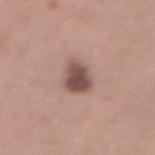biopsy status: total-body-photography surveillance lesion; no biopsy | automated lesion analysis: a mean CIELAB color near L≈50 a*≈19 b*≈22, roughly 14 lightness units darker than nearby skin, and a normalized lesion–skin contrast near 10 | patient: female, in their 30s | image source: ~15 mm crop, total-body skin-cancer survey | diameter: ≈4.5 mm | tile lighting: white-light | anatomic site: the lower back.